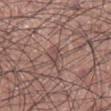Q: Is there a histopathology result?
A: total-body-photography surveillance lesion; no biopsy
Q: What kind of image is this?
A: ~15 mm crop, total-body skin-cancer survey
Q: How was the tile lit?
A: white-light
Q: Where on the body is the lesion?
A: the right lower leg
Q: Automated lesion metrics?
A: a footprint of about 2.5 mm²; border irregularity of about 2.5 on a 0–10 scale, internal color variation of about 0 on a 0–10 scale, and peripheral color asymmetry of about 0; lesion-presence confidence of about 85/100
Q: Who is the patient?
A: male, aged 23 to 27